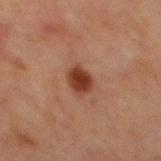No biopsy was performed on this lesion — it was imaged during a full skin examination and was not determined to be concerning.
Captured under cross-polarized illumination.
The subject is a male aged approximately 65.
A 15 mm close-up extracted from a 3D total-body photography capture.
Measured at roughly 3.5 mm in maximum diameter.
On the abdomen.